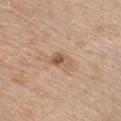* follow-up — no biopsy performed (imaged during a skin exam)
* imaging modality — ~15 mm crop, total-body skin-cancer survey
* patient — female, in their mid-40s
* body site — the chest
* TBP lesion metrics — a lesion–skin lightness drop of about 10
* lighting — white-light